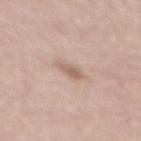No biopsy was performed on this lesion — it was imaged during a full skin examination and was not determined to be concerning. This image is a 15 mm lesion crop taken from a total-body photograph. A female patient, aged 58 to 62. On the back.